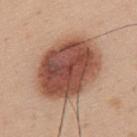Clinical impression:
Imaged during a routine full-body skin examination; the lesion was not biopsied and no histopathology is available.
Background:
A male subject, aged approximately 35. Cropped from a total-body skin-imaging series; the visible field is about 15 mm. On the back.